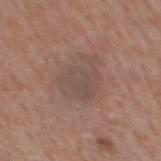notes — imaged on a skin check; not biopsied | illumination — white-light illumination | image-analysis metrics — a shape eccentricity near 0.7 and a symmetry-axis asymmetry near 0.35; a border-irregularity rating of about 5/10, internal color variation of about 2 on a 0–10 scale, and radial color variation of about 0.5 | site — the mid back | lesion size — ≈5.5 mm | image — total-body-photography crop, ~15 mm field of view | patient — male, aged 53–57.